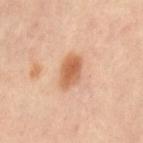Acquisition and patient details: The subject is a female aged 38–42. Captured under cross-polarized illumination. The lesion is located on the lower back. A 15 mm close-up tile from a total-body photography series done for melanoma screening. An algorithmic analysis of the crop reported a footprint of about 7.5 mm² and a shape eccentricity near 0.8. It also reported a lesion-to-skin contrast of about 8.5 (normalized; higher = more distinct). The software also gave an automated nevus-likeness rating near 95 out of 100. The lesion's longest dimension is about 4 mm.